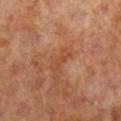{
  "biopsy_status": "not biopsied; imaged during a skin examination",
  "lesion_size": {
    "long_diameter_mm_approx": 3.0
  },
  "patient": {
    "sex": "female",
    "age_approx": 65
  },
  "lighting": "cross-polarized",
  "site": "right lower leg",
  "image": {
    "source": "total-body photography crop",
    "field_of_view_mm": 15
  }
}